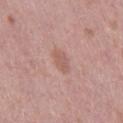Clinical impression: The lesion was photographed on a routine skin check and not biopsied; there is no pathology result. Clinical summary: About 2.5 mm across. A lesion tile, about 15 mm wide, cut from a 3D total-body photograph. Imaged with white-light lighting. The subject is a female aged around 40. On the right thigh.